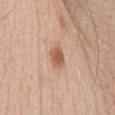Q: Was this lesion biopsied?
A: no biopsy performed (imaged during a skin exam)
Q: What is the imaging modality?
A: ~15 mm tile from a whole-body skin photo
Q: Patient demographics?
A: male, aged 58–62
Q: What lighting was used for the tile?
A: white-light
Q: What is the lesion's diameter?
A: ≈3 mm
Q: What is the anatomic site?
A: the abdomen
Q: What did automated image analysis measure?
A: a lesion–skin lightness drop of about 12 and a lesion-to-skin contrast of about 8 (normalized; higher = more distinct); a border-irregularity index near 1.5/10, a within-lesion color-variation index near 2/10, and radial color variation of about 0.5; an automated nevus-likeness rating near 95 out of 100 and a detector confidence of about 100 out of 100 that the crop contains a lesion